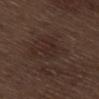Impression: Recorded during total-body skin imaging; not selected for excision or biopsy. Acquisition and patient details: A male patient, aged approximately 70. Captured under white-light illumination. The lesion's longest dimension is about 3 mm. Automated image analysis of the tile measured a lesion area of about 4 mm² and an outline eccentricity of about 0.85 (0 = round, 1 = elongated). It also reported a lesion–skin lightness drop of about 4 and a normalized lesion–skin contrast near 5. The software also gave a classifier nevus-likeness of about 0/100 and lesion-presence confidence of about 100/100. A lesion tile, about 15 mm wide, cut from a 3D total-body photograph. From the right thigh.